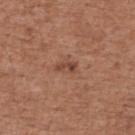Clinical impression:
Part of a total-body skin-imaging series; this lesion was reviewed on a skin check and was not flagged for biopsy.
Clinical summary:
On the upper back. The lesion's longest dimension is about 2.5 mm. An algorithmic analysis of the crop reported a color-variation rating of about 1.5/10 and a peripheral color-asymmetry measure near 0.5. A region of skin cropped from a whole-body photographic capture, roughly 15 mm wide. The tile uses white-light illumination. The patient is a male about 75 years old.